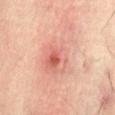The lesion-visualizer software estimated an area of roughly 37 mm² and a symmetry-axis asymmetry near 0.25.
Located on the front of the torso.
About 11.5 mm across.
A male subject, aged 58 to 62.
A region of skin cropped from a whole-body photographic capture, roughly 15 mm wide.
Captured under cross-polarized illumination.